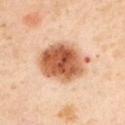Q: Was a biopsy performed?
A: catalogued during a skin exam; not biopsied
Q: Automated lesion metrics?
A: an area of roughly 23 mm², a shape eccentricity near 0.65, and a symmetry-axis asymmetry near 0.15; border irregularity of about 1.5 on a 0–10 scale, internal color variation of about 8.5 on a 0–10 scale, and a peripheral color-asymmetry measure near 2.5
Q: What kind of image is this?
A: 15 mm crop, total-body photography
Q: What lighting was used for the tile?
A: cross-polarized
Q: Who is the patient?
A: male, in their mid-50s
Q: Lesion location?
A: the chest